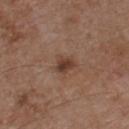No biopsy was performed on this lesion — it was imaged during a full skin examination and was not determined to be concerning.
This image is a 15 mm lesion crop taken from a total-body photograph.
A male subject roughly 55 years of age.
The lesion is located on the front of the torso.